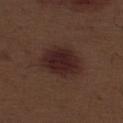<tbp_lesion>
  <biopsy_status>not biopsied; imaged during a skin examination</biopsy_status>
  <image>
    <source>total-body photography crop</source>
    <field_of_view_mm>15</field_of_view_mm>
  </image>
  <site>left thigh</site>
  <automated_metrics>
    <area_mm2_approx>15.0</area_mm2_approx>
    <eccentricity>0.75</eccentricity>
    <shape_asymmetry>0.2</shape_asymmetry>
    <vs_skin_contrast_norm>10.5</vs_skin_contrast_norm>
    <nevus_likeness_0_100>95</nevus_likeness_0_100>
    <lesion_detection_confidence_0_100>100</lesion_detection_confidence_0_100>
  </automated_metrics>
  <lighting>white-light</lighting>
  <lesion_size>
    <long_diameter_mm_approx>5.5</long_diameter_mm_approx>
  </lesion_size>
  <patient>
    <sex>male</sex>
    <age_approx>70</age_approx>
  </patient>
</tbp_lesion>